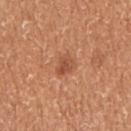| feature | finding |
|---|---|
| notes | catalogued during a skin exam; not biopsied |
| site | the right upper arm |
| lesion diameter | ≈2.5 mm |
| patient | female, aged around 35 |
| illumination | white-light illumination |
| image source | ~15 mm crop, total-body skin-cancer survey |
| automated metrics | a lesion area of about 4 mm², an eccentricity of roughly 0.65, and a shape-asymmetry score of about 0.25 (0 = symmetric); a lesion–skin lightness drop of about 9 and a lesion-to-skin contrast of about 6.5 (normalized; higher = more distinct); an automated nevus-likeness rating near 60 out of 100 and lesion-presence confidence of about 100/100 |